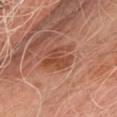Q: Was this lesion biopsied?
A: imaged on a skin check; not biopsied
Q: How was this image acquired?
A: total-body-photography crop, ~15 mm field of view
Q: What are the patient's age and sex?
A: male, aged 48 to 52
Q: Where on the body is the lesion?
A: the chest